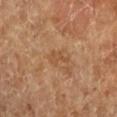Part of a total-body skin-imaging series; this lesion was reviewed on a skin check and was not flagged for biopsy. The recorded lesion diameter is about 2.5 mm. The subject is a female in their 70s. Automated tile analysis of the lesion measured a footprint of about 2.5 mm² and two-axis asymmetry of about 0.6. It also reported an average lesion color of about L≈48 a*≈20 b*≈34 (CIELAB), about 6 CIELAB-L* units darker than the surrounding skin, and a normalized lesion–skin contrast near 5. The software also gave border irregularity of about 7 on a 0–10 scale and a within-lesion color-variation index near 0/10. And it measured lesion-presence confidence of about 100/100. From the right forearm. A 15 mm close-up extracted from a 3D total-body photography capture. Imaged with cross-polarized lighting.